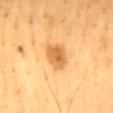Captured during whole-body skin photography for melanoma surveillance; the lesion was not biopsied. The lesion is on the mid back. Imaged with cross-polarized lighting. A male patient, approximately 60 years of age. A 15 mm close-up tile from a total-body photography series done for melanoma screening.